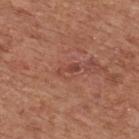Notes:
* workup: catalogued during a skin exam; not biopsied
* site: the upper back
* imaging modality: 15 mm crop, total-body photography
* automated lesion analysis: an outline eccentricity of about 0.95 (0 = round, 1 = elongated) and a symmetry-axis asymmetry near 0.4; a mean CIELAB color near L≈44 a*≈27 b*≈28 and a lesion-to-skin contrast of about 6 (normalized; higher = more distinct); a border-irregularity rating of about 5/10, a within-lesion color-variation index near 0/10, and a peripheral color-asymmetry measure near 0; a nevus-likeness score of about 0/100 and a detector confidence of about 95 out of 100 that the crop contains a lesion
* subject: male, in their mid-60s
* lesion size: about 2.5 mm